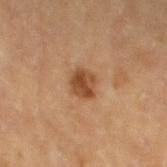Part of a total-body skin-imaging series; this lesion was reviewed on a skin check and was not flagged for biopsy. A male patient, roughly 85 years of age or older. Captured under cross-polarized illumination. A 15 mm close-up tile from a total-body photography series done for melanoma screening. The lesion is located on the left thigh. The recorded lesion diameter is about 3 mm. The total-body-photography lesion software estimated a footprint of about 5.5 mm², an eccentricity of roughly 0.6, and a shape-asymmetry score of about 0.2 (0 = symmetric). It also reported a lesion color around L≈40 a*≈20 b*≈31 in CIELAB and a lesion-to-skin contrast of about 9 (normalized; higher = more distinct).